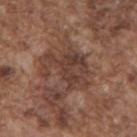The lesion was photographed on a routine skin check and not biopsied; there is no pathology result.